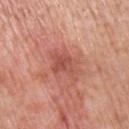The lesion was tiled from a total-body skin photograph and was not biopsied. Cropped from a total-body skin-imaging series; the visible field is about 15 mm. Imaged with white-light lighting. A male subject, roughly 70 years of age. On the right upper arm.